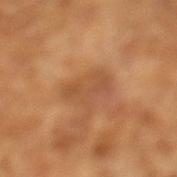Assessment:
The lesion was photographed on a routine skin check and not biopsied; there is no pathology result.
Context:
The total-body-photography lesion software estimated an area of roughly 6.5 mm², an eccentricity of roughly 0.85, and two-axis asymmetry of about 0.45. The software also gave a nevus-likeness score of about 0/100 and a lesion-detection confidence of about 100/100. Imaged with cross-polarized lighting. A male patient, aged 63–67. Approximately 4 mm at its widest. A region of skin cropped from a whole-body photographic capture, roughly 15 mm wide.